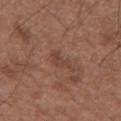{"biopsy_status": "not biopsied; imaged during a skin examination", "lesion_size": {"long_diameter_mm_approx": 2.5}, "automated_metrics": {"cielab_L": 42, "cielab_a": 20, "cielab_b": 25, "vs_skin_darker_L": 6.0, "vs_skin_contrast_norm": 5.0, "border_irregularity_0_10": 5.5, "peripheral_color_asymmetry": 0.0}, "image": {"source": "total-body photography crop", "field_of_view_mm": 15}, "site": "left forearm", "patient": {"sex": "male", "age_approx": 50}, "lighting": "white-light"}